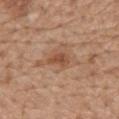On the mid back.
A male subject roughly 70 years of age.
A region of skin cropped from a whole-body photographic capture, roughly 15 mm wide.